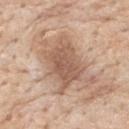biopsy status=no biopsy performed (imaged during a skin exam) | lesion size=~7.5 mm (longest diameter) | location=the upper back | lighting=white-light | TBP lesion metrics=an area of roughly 24 mm² and an eccentricity of roughly 0.75; a within-lesion color-variation index near 3.5/10 and a peripheral color-asymmetry measure near 1; a classifier nevus-likeness of about 5/100 and a lesion-detection confidence of about 100/100 | image source=~15 mm crop, total-body skin-cancer survey | patient=male, aged approximately 85 or older.